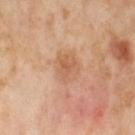Clinical impression: The lesion was tiled from a total-body skin photograph and was not biopsied. Acquisition and patient details: The subject is a female in their mid-50s. The total-body-photography lesion software estimated a border-irregularity index near 2/10 and a peripheral color-asymmetry measure near 1. And it measured an automated nevus-likeness rating near 0 out of 100 and a detector confidence of about 100 out of 100 that the crop contains a lesion. A region of skin cropped from a whole-body photographic capture, roughly 15 mm wide. The lesion is located on the right thigh.